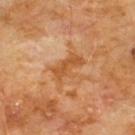Captured during whole-body skin photography for melanoma surveillance; the lesion was not biopsied.
Located on the front of the torso.
A 15 mm crop from a total-body photograph taken for skin-cancer surveillance.
A male patient, aged approximately 60.